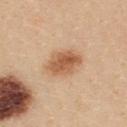The lesion was tiled from a total-body skin photograph and was not biopsied. Imaged with white-light lighting. The subject is a female in their mid- to late 20s. This image is a 15 mm lesion crop taken from a total-body photograph. The lesion-visualizer software estimated a lesion area of about 10 mm² and a symmetry-axis asymmetry near 0.15. And it measured a classifier nevus-likeness of about 100/100 and lesion-presence confidence of about 100/100. The lesion is located on the upper back. About 4.5 mm across.